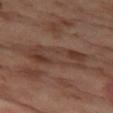The lesion was tiled from a total-body skin photograph and was not biopsied. A 15 mm close-up extracted from a 3D total-body photography capture. A female subject roughly 55 years of age. Imaged with cross-polarized lighting. From the left thigh. Automated tile analysis of the lesion measured a border-irregularity index near 3/10, a color-variation rating of about 5.5/10, and a peripheral color-asymmetry measure near 1.5.